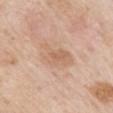Q: Was a biopsy performed?
A: total-body-photography surveillance lesion; no biopsy
Q: What are the patient's age and sex?
A: male, aged approximately 85 or older
Q: Where on the body is the lesion?
A: the mid back
Q: What lighting was used for the tile?
A: white-light
Q: What is the imaging modality?
A: 15 mm crop, total-body photography